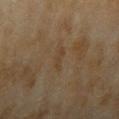Imaged during a routine full-body skin examination; the lesion was not biopsied and no histopathology is available.
Automated image analysis of the tile measured a footprint of about 2.5 mm² and a shape eccentricity near 0.9. And it measured a border-irregularity rating of about 5.5/10, a within-lesion color-variation index near 0/10, and radial color variation of about 0.
Cropped from a total-body skin-imaging series; the visible field is about 15 mm.
The recorded lesion diameter is about 2.5 mm.
The subject is a female in their 60s.
On the right upper arm.
The tile uses cross-polarized illumination.